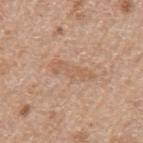| feature | finding |
|---|---|
| workup | imaged on a skin check; not biopsied |
| illumination | white-light |
| lesion diameter | ≈5 mm |
| image | ~15 mm tile from a whole-body skin photo |
| body site | the arm |
| automated metrics | a footprint of about 7 mm², an eccentricity of roughly 0.95, and a symmetry-axis asymmetry near 0.25; border irregularity of about 4 on a 0–10 scale, internal color variation of about 1.5 on a 0–10 scale, and a peripheral color-asymmetry measure near 0.5 |
| patient | female, in their 70s |